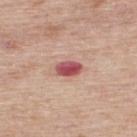workup: no biopsy performed (imaged during a skin exam) | illumination: white-light illumination | lesion diameter: ~3 mm (longest diameter) | location: the upper back | patient: male, aged 73–77 | TBP lesion metrics: an area of roughly 4.5 mm², an eccentricity of roughly 0.8, and a symmetry-axis asymmetry near 0.2; a lesion color around L≈52 a*≈33 b*≈23 in CIELAB, a lesion–skin lightness drop of about 17, and a lesion-to-skin contrast of about 11 (normalized; higher = more distinct); a border-irregularity rating of about 2/10 and a color-variation rating of about 5/10 | image source: total-body-photography crop, ~15 mm field of view.